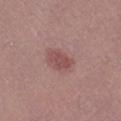Assessment:
Captured during whole-body skin photography for melanoma surveillance; the lesion was not biopsied.
Background:
Cropped from a whole-body photographic skin survey; the tile spans about 15 mm. From the left leg. A female subject, aged approximately 35. The lesion's longest dimension is about 3 mm. This is a white-light tile.